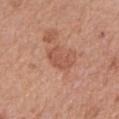follow-up — imaged on a skin check; not biopsied
image source — ~15 mm tile from a whole-body skin photo
location — the right forearm
size — ≈3.5 mm
patient — female, approximately 60 years of age
automated metrics — a footprint of about 5.5 mm² and a shape-asymmetry score of about 0.35 (0 = symmetric); a lesion color around L≈54 a*≈25 b*≈31 in CIELAB, about 8 CIELAB-L* units darker than the surrounding skin, and a normalized border contrast of about 5.5; a border-irregularity index near 4.5/10 and internal color variation of about 1 on a 0–10 scale; an automated nevus-likeness rating near 10 out of 100